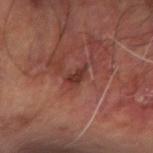Q: Was this lesion biopsied?
A: total-body-photography surveillance lesion; no biopsy
Q: Lesion location?
A: the left arm
Q: How was this image acquired?
A: total-body-photography crop, ~15 mm field of view
Q: Patient demographics?
A: male, in their 60s